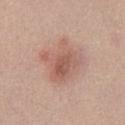Imaged with white-light lighting.
Cropped from a whole-body photographic skin survey; the tile spans about 15 mm.
From the left thigh.
The subject is a female roughly 20 years of age.